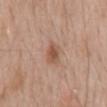Part of a total-body skin-imaging series; this lesion was reviewed on a skin check and was not flagged for biopsy.
The lesion is located on the mid back.
A 15 mm close-up extracted from a 3D total-body photography capture.
The patient is a male aged 58 to 62.